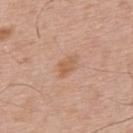workup: no biopsy performed (imaged during a skin exam); location: the upper back; imaging modality: ~15 mm tile from a whole-body skin photo; lesion size: ~3 mm (longest diameter); patient: male, aged 63 to 67.